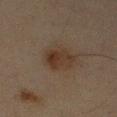* follow-up: imaged on a skin check; not biopsied
* lighting: cross-polarized
* site: the right upper arm
* acquisition: 15 mm crop, total-body photography
* subject: male, approximately 65 years of age
* size: ~4 mm (longest diameter)